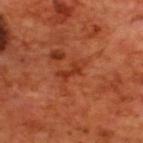Assessment:
Part of a total-body skin-imaging series; this lesion was reviewed on a skin check and was not flagged for biopsy.
Clinical summary:
A male patient approximately 70 years of age. Cropped from a whole-body photographic skin survey; the tile spans about 15 mm. From the upper back. The recorded lesion diameter is about 3 mm. Automated tile analysis of the lesion measured a lesion area of about 4 mm² and a symmetry-axis asymmetry near 0.4.